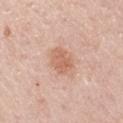Q: Was a biopsy performed?
A: catalogued during a skin exam; not biopsied
Q: How large is the lesion?
A: ≈4 mm
Q: Lesion location?
A: the left upper arm
Q: What is the imaging modality?
A: ~15 mm tile from a whole-body skin photo
Q: What are the patient's age and sex?
A: male, approximately 60 years of age